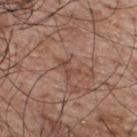Q: Was this lesion biopsied?
A: catalogued during a skin exam; not biopsied
Q: Where on the body is the lesion?
A: the upper back
Q: How was the tile lit?
A: white-light illumination
Q: Patient demographics?
A: male, aged approximately 70
Q: How was this image acquired?
A: ~15 mm crop, total-body skin-cancer survey